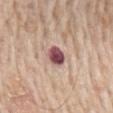<case>
<biopsy_status>not biopsied; imaged during a skin examination</biopsy_status>
</case>